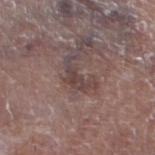notes: catalogued during a skin exam; not biopsied | image: ~15 mm tile from a whole-body skin photo | subject: male, approximately 80 years of age | body site: the leg.